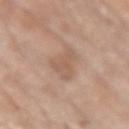Q: Was this lesion biopsied?
A: no biopsy performed (imaged during a skin exam)
Q: What is the anatomic site?
A: the right upper arm
Q: How large is the lesion?
A: ~4.5 mm (longest diameter)
Q: What did automated image analysis measure?
A: an eccentricity of roughly 0.9 and two-axis asymmetry of about 0.5; a mean CIELAB color near L≈58 a*≈18 b*≈29, a lesion–skin lightness drop of about 7, and a normalized border contrast of about 5; a border-irregularity index near 5/10, a within-lesion color-variation index near 2/10, and a peripheral color-asymmetry measure near 0.5; a classifier nevus-likeness of about 0/100 and lesion-presence confidence of about 100/100
Q: How was the tile lit?
A: white-light illumination
Q: What kind of image is this?
A: 15 mm crop, total-body photography
Q: Patient demographics?
A: male, aged 78 to 82